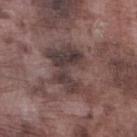Imaged with white-light lighting. Cropped from a whole-body photographic skin survey; the tile spans about 15 mm. Approximately 6.5 mm at its widest. The patient is a male aged approximately 75. On the left lower leg.